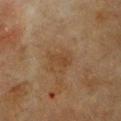Q: Is there a histopathology result?
A: total-body-photography surveillance lesion; no biopsy
Q: Where on the body is the lesion?
A: the chest
Q: What is the lesion's diameter?
A: ~2.5 mm (longest diameter)
Q: Patient demographics?
A: female, approximately 55 years of age
Q: What kind of image is this?
A: total-body-photography crop, ~15 mm field of view
Q: How was the tile lit?
A: cross-polarized illumination
Q: Automated lesion metrics?
A: a footprint of about 2.5 mm², an outline eccentricity of about 0.85 (0 = round, 1 = elongated), and two-axis asymmetry of about 0.3; an average lesion color of about L≈34 a*≈16 b*≈29 (CIELAB), about 5 CIELAB-L* units darker than the surrounding skin, and a lesion-to-skin contrast of about 6 (normalized; higher = more distinct); border irregularity of about 3 on a 0–10 scale; a nevus-likeness score of about 0/100